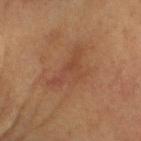notes: catalogued during a skin exam; not biopsied
subject: male, in their mid-60s
lesion diameter: about 6 mm
location: the head or neck
image source: 15 mm crop, total-body photography
TBP lesion metrics: about 6 CIELAB-L* units darker than the surrounding skin and a normalized border contrast of about 5; a classifier nevus-likeness of about 20/100
illumination: cross-polarized illumination